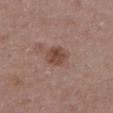Assessment: Recorded during total-body skin imaging; not selected for excision or biopsy. Image and clinical context: Cropped from a whole-body photographic skin survey; the tile spans about 15 mm. Longest diameter approximately 3 mm. From the upper back. This is a white-light tile. A female subject aged approximately 65.